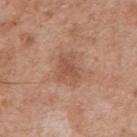Captured during whole-body skin photography for melanoma surveillance; the lesion was not biopsied.
Longest diameter approximately 3 mm.
A lesion tile, about 15 mm wide, cut from a 3D total-body photograph.
The patient is a male aged 48–52.
On the right upper arm.